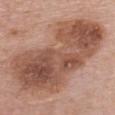  patient:
    sex: female
    age_approx: 60
  lesion_size:
    long_diameter_mm_approx: 13.5
  site: chest
  image:
    source: total-body photography crop
    field_of_view_mm: 15